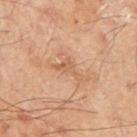The lesion was tiled from a total-body skin photograph and was not biopsied.
An algorithmic analysis of the crop reported a mean CIELAB color near L≈57 a*≈21 b*≈34, a lesion–skin lightness drop of about 8, and a normalized lesion–skin contrast near 5.5. The software also gave a border-irregularity index near 7.5/10 and radial color variation of about 0. It also reported an automated nevus-likeness rating near 0 out of 100.
The lesion is on the right upper arm.
A male subject, aged 63 to 67.
Measured at roughly 3.5 mm in maximum diameter.
This is a cross-polarized tile.
A 15 mm crop from a total-body photograph taken for skin-cancer surveillance.